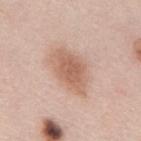Assessment:
The lesion was tiled from a total-body skin photograph and was not biopsied.
Context:
An algorithmic analysis of the crop reported a lesion area of about 15 mm² and a shape eccentricity near 0.8. It also reported an average lesion color of about L≈62 a*≈20 b*≈29 (CIELAB), a lesion–skin lightness drop of about 11, and a normalized border contrast of about 7.5. The software also gave a nevus-likeness score of about 90/100 and lesion-presence confidence of about 100/100. Longest diameter approximately 6 mm. The patient is a male aged 43 to 47. Captured under white-light illumination. Cropped from a whole-body photographic skin survey; the tile spans about 15 mm. Located on the mid back.